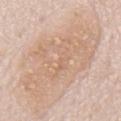Impression:
The lesion was tiled from a total-body skin photograph and was not biopsied.
Background:
A male patient, roughly 75 years of age. On the chest. Imaged with white-light lighting. A 15 mm close-up tile from a total-body photography series done for melanoma screening. About 11 mm across. The total-body-photography lesion software estimated a symmetry-axis asymmetry near 0.25. The software also gave a mean CIELAB color near L≈68 a*≈17 b*≈30. The analysis additionally found internal color variation of about 4 on a 0–10 scale and a peripheral color-asymmetry measure near 1.5.